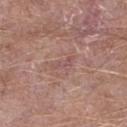workup: no biopsy performed (imaged during a skin exam)
body site: the left lower leg
image: ~15 mm crop, total-body skin-cancer survey
image-analysis metrics: a lesion area of about 3 mm², an outline eccentricity of about 0.95 (0 = round, 1 = elongated), and two-axis asymmetry of about 0.45; an average lesion color of about L≈52 a*≈21 b*≈21 (CIELAB), roughly 6 lightness units darker than nearby skin, and a lesion-to-skin contrast of about 5 (normalized; higher = more distinct); a border-irregularity index near 5.5/10 and a color-variation rating of about 0/10; an automated nevus-likeness rating near 0 out of 100
patient: male, aged around 55
lesion diameter: ~3.5 mm (longest diameter)
tile lighting: white-light illumination